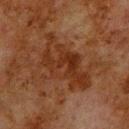Impression:
Captured during whole-body skin photography for melanoma surveillance; the lesion was not biopsied.
Background:
About 8 mm across. The lesion is located on the upper back. A male patient aged around 80. The tile uses cross-polarized illumination. This image is a 15 mm lesion crop taken from a total-body photograph.